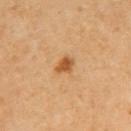<tbp_lesion>
  <biopsy_status>not biopsied; imaged during a skin examination</biopsy_status>
  <lighting>cross-polarized</lighting>
  <site>upper back</site>
  <lesion_size>
    <long_diameter_mm_approx>2.5</long_diameter_mm_approx>
  </lesion_size>
  <patient>
    <sex>female</sex>
    <age_approx>65</age_approx>
  </patient>
  <image>
    <source>total-body photography crop</source>
    <field_of_view_mm>15</field_of_view_mm>
  </image>
  <automated_metrics>
    <cielab_L>54</cielab_L>
    <cielab_a>23</cielab_a>
    <cielab_b>41</cielab_b>
    <vs_skin_contrast_norm>8.5</vs_skin_contrast_norm>
    <border_irregularity_0_10>2.5</border_irregularity_0_10>
    <color_variation_0_10>2.0</color_variation_0_10>
    <peripheral_color_asymmetry>1.0</peripheral_color_asymmetry>
  </automated_metrics>
</tbp_lesion>